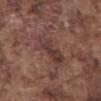The lesion was photographed on a routine skin check and not biopsied; there is no pathology result. A male subject, aged 73 to 77. A 15 mm close-up tile from a total-body photography series done for melanoma screening. The total-body-photography lesion software estimated border irregularity of about 4 on a 0–10 scale and a peripheral color-asymmetry measure near 1.5. It also reported a nevus-likeness score of about 0/100 and a detector confidence of about 60 out of 100 that the crop contains a lesion. Captured under white-light illumination. On the abdomen. Measured at roughly 5 mm in maximum diameter.